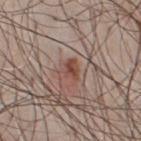<lesion>
  <biopsy_status>not biopsied; imaged during a skin examination</biopsy_status>
  <lighting>white-light</lighting>
  <patient>
    <sex>male</sex>
    <age_approx>50</age_approx>
  </patient>
  <lesion_size>
    <long_diameter_mm_approx>2.5</long_diameter_mm_approx>
  </lesion_size>
  <automated_metrics>
    <area_mm2_approx>4.0</area_mm2_approx>
    <vs_skin_darker_L>9.0</vs_skin_darker_L>
    <vs_skin_contrast_norm>7.5</vs_skin_contrast_norm>
    <color_variation_0_10>3.5</color_variation_0_10>
    <peripheral_color_asymmetry>1.0</peripheral_color_asymmetry>
  </automated_metrics>
  <image>
    <source>total-body photography crop</source>
    <field_of_view_mm>15</field_of_view_mm>
  </image>
  <site>chest</site>
</lesion>